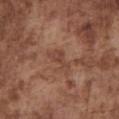Image and clinical context: A male subject in their mid-70s. Located on the chest. Imaged with white-light lighting. A region of skin cropped from a whole-body photographic capture, roughly 15 mm wide. About 3.5 mm across. The total-body-photography lesion software estimated a footprint of about 4 mm² and two-axis asymmetry of about 0.45. The analysis additionally found a mean CIELAB color near L≈43 a*≈21 b*≈27.